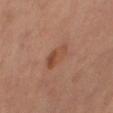A female patient aged 63–67. A lesion tile, about 15 mm wide, cut from a 3D total-body photograph. Approximately 4 mm at its widest. The lesion is located on the right thigh. Captured under cross-polarized illumination.